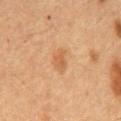{"biopsy_status": "not biopsied; imaged during a skin examination", "image": {"source": "total-body photography crop", "field_of_view_mm": 15}, "site": "back", "lesion_size": {"long_diameter_mm_approx": 3.0}, "lighting": "cross-polarized", "patient": {"sex": "male", "age_approx": 65}}